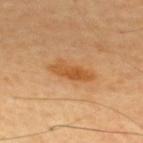No biopsy was performed on this lesion — it was imaged during a full skin examination and was not determined to be concerning.
Approximately 5 mm at its widest.
The total-body-photography lesion software estimated a mean CIELAB color near L≈55 a*≈23 b*≈43 and a normalized border contrast of about 7.5. It also reported a peripheral color-asymmetry measure near 1. It also reported an automated nevus-likeness rating near 60 out of 100 and lesion-presence confidence of about 100/100.
A region of skin cropped from a whole-body photographic capture, roughly 15 mm wide.
A male subject aged approximately 65.
The lesion is on the upper back.
The tile uses cross-polarized illumination.